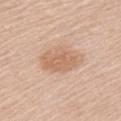Clinical impression: Part of a total-body skin-imaging series; this lesion was reviewed on a skin check and was not flagged for biopsy.